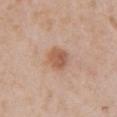follow-up — catalogued during a skin exam; not biopsied | image source — ~15 mm tile from a whole-body skin photo | size — ~2.5 mm (longest diameter) | location — the front of the torso | patient — female, roughly 60 years of age.